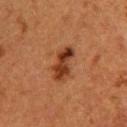The lesion was tiled from a total-body skin photograph and was not biopsied. From the back. A female patient, aged around 40. A 15 mm close-up tile from a total-body photography series done for melanoma screening.